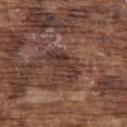• follow-up · imaged on a skin check; not biopsied
• tile lighting · white-light illumination
• body site · the upper back
• lesion diameter · ~4.5 mm (longest diameter)
• subject · male, aged around 75
• image · 15 mm crop, total-body photography